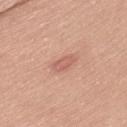Assessment: Captured during whole-body skin photography for melanoma surveillance; the lesion was not biopsied. Context: Captured under white-light illumination. A female patient in their 50s. Longest diameter approximately 3 mm. A close-up tile cropped from a whole-body skin photograph, about 15 mm across. Located on the left thigh. Automated image analysis of the tile measured an area of roughly 3.5 mm², a shape eccentricity near 0.85, and two-axis asymmetry of about 0.25.